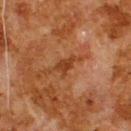Notes:
- follow-up: imaged on a skin check; not biopsied
- anatomic site: the upper back
- subject: male, approximately 80 years of age
- imaging modality: 15 mm crop, total-body photography